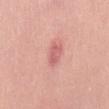| feature | finding |
|---|---|
| notes | catalogued during a skin exam; not biopsied |
| tile lighting | white-light |
| subject | male, aged approximately 30 |
| image | ~15 mm tile from a whole-body skin photo |